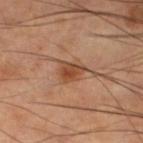Captured during whole-body skin photography for melanoma surveillance; the lesion was not biopsied.
Imaged with cross-polarized lighting.
Cropped from a total-body skin-imaging series; the visible field is about 15 mm.
A male patient, about 70 years old.
An algorithmic analysis of the crop reported an automated nevus-likeness rating near 80 out of 100 and a lesion-detection confidence of about 100/100.
Located on the left lower leg.